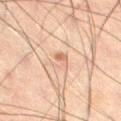Notes:
• lesion diameter: ~2.5 mm (longest diameter)
• anatomic site: the left leg
• illumination: cross-polarized illumination
• subject: male, aged 58–62
• image source: ~15 mm crop, total-body skin-cancer survey